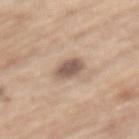notes=no biopsy performed (imaged during a skin exam) | location=the back | image source=~15 mm tile from a whole-body skin photo | subject=male, roughly 70 years of age | illumination=white-light | automated metrics=an area of roughly 5.5 mm² and a symmetry-axis asymmetry near 0.15; roughly 14 lightness units darker than nearby skin; a border-irregularity index near 1.5/10 and peripheral color asymmetry of about 0.5; a nevus-likeness score of about 65/100 and lesion-presence confidence of about 100/100 | size=about 3 mm.